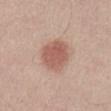Captured during whole-body skin photography for melanoma surveillance; the lesion was not biopsied. Located on the abdomen. The lesion's longest dimension is about 4.5 mm. This is a white-light tile. A male patient aged approximately 40. A 15 mm crop from a total-body photograph taken for skin-cancer surveillance.